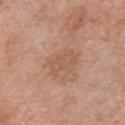Case summary:
• notes — imaged on a skin check; not biopsied
• acquisition — total-body-photography crop, ~15 mm field of view
• site — the chest
• subject — male, about 70 years old